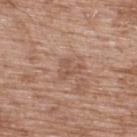biopsy status: total-body-photography surveillance lesion; no biopsy
location: the upper back
TBP lesion metrics: a border-irregularity rating of about 5.5/10, a within-lesion color-variation index near 1/10, and peripheral color asymmetry of about 0.5; a nevus-likeness score of about 0/100 and lesion-presence confidence of about 100/100
lighting: white-light illumination
subject: male, aged approximately 50
lesion size: ~3 mm (longest diameter)
imaging modality: total-body-photography crop, ~15 mm field of view